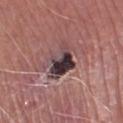The lesion was tiled from a total-body skin photograph and was not biopsied. The recorded lesion diameter is about 4 mm. The lesion is located on the left lower leg. A male subject, in their mid-60s. Captured under white-light illumination. A roughly 15 mm field-of-view crop from a total-body skin photograph.